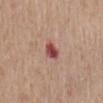Part of a total-body skin-imaging series; this lesion was reviewed on a skin check and was not flagged for biopsy. Cropped from a total-body skin-imaging series; the visible field is about 15 mm. Measured at roughly 2.5 mm in maximum diameter. The patient is a male in their mid- to late 60s. An algorithmic analysis of the crop reported an outline eccentricity of about 0.65 (0 = round, 1 = elongated) and two-axis asymmetry of about 0.2. The software also gave an average lesion color of about L≈48 a*≈29 b*≈23 (CIELAB), about 15 CIELAB-L* units darker than the surrounding skin, and a lesion-to-skin contrast of about 10.5 (normalized; higher = more distinct). The lesion is located on the lower back.